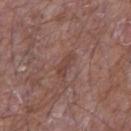{"patient": {"sex": "male", "age_approx": 65}, "image": {"source": "total-body photography crop", "field_of_view_mm": 15}, "automated_metrics": {"area_mm2_approx": 3.0, "shape_asymmetry": 0.35, "border_irregularity_0_10": 4.0, "color_variation_0_10": 0.0, "peripheral_color_asymmetry": 0.0, "nevus_likeness_0_100": 0, "lesion_detection_confidence_0_100": 100}, "lighting": "white-light", "site": "right upper arm", "lesion_size": {"long_diameter_mm_approx": 3.0}}